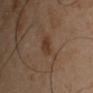Impression:
This lesion was catalogued during total-body skin photography and was not selected for biopsy.
Image and clinical context:
A roughly 15 mm field-of-view crop from a total-body skin photograph. A male subject, aged around 45. Located on the left upper arm.